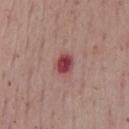Case summary:
• biopsy status · catalogued during a skin exam; not biopsied
• automated lesion analysis · a lesion color around L≈44 a*≈31 b*≈20 in CIELAB, about 14 CIELAB-L* units darker than the surrounding skin, and a normalized border contrast of about 10.5
• image · total-body-photography crop, ~15 mm field of view
• subject · male, aged 68–72
• location · the chest
• lighting · white-light illumination
• lesion size · about 2.5 mm